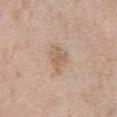{
  "biopsy_status": "not biopsied; imaged during a skin examination",
  "patient": {
    "sex": "male",
    "age_approx": 50
  },
  "image": {
    "source": "total-body photography crop",
    "field_of_view_mm": 15
  },
  "site": "front of the torso"
}